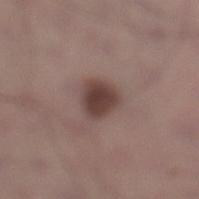Part of a total-body skin-imaging series; this lesion was reviewed on a skin check and was not flagged for biopsy. Captured under white-light illumination. A roughly 15 mm field-of-view crop from a total-body skin photograph. A male patient, in their mid-30s. The lesion is located on the leg.